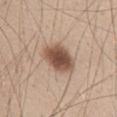Recorded during total-body skin imaging; not selected for excision or biopsy.
The subject is a male about 45 years old.
Imaged with white-light lighting.
Approximately 4.5 mm at its widest.
This image is a 15 mm lesion crop taken from a total-body photograph.
From the abdomen.
The lesion-visualizer software estimated an area of roughly 12 mm², an eccentricity of roughly 0.75, and a symmetry-axis asymmetry near 0.2. It also reported an average lesion color of about L≈52 a*≈18 b*≈27 (CIELAB) and a lesion-to-skin contrast of about 11 (normalized; higher = more distinct). The analysis additionally found border irregularity of about 1.5 on a 0–10 scale, a within-lesion color-variation index near 4.5/10, and peripheral color asymmetry of about 1.